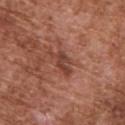This lesion was catalogued during total-body skin photography and was not selected for biopsy. Imaged with white-light lighting. Approximately 4 mm at its widest. A close-up tile cropped from a whole-body skin photograph, about 15 mm across. The patient is a male approximately 75 years of age. The lesion is located on the back.